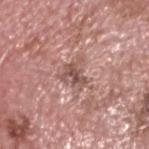No biopsy was performed on this lesion — it was imaged during a full skin examination and was not determined to be concerning. Measured at roughly 3.5 mm in maximum diameter. Imaged with white-light lighting. An algorithmic analysis of the crop reported an average lesion color of about L≈53 a*≈21 b*≈23 (CIELAB), about 10 CIELAB-L* units darker than the surrounding skin, and a normalized lesion–skin contrast near 7. The software also gave a nevus-likeness score of about 0/100 and a lesion-detection confidence of about 55/100. A male patient, aged approximately 75. A 15 mm crop from a total-body photograph taken for skin-cancer surveillance. The lesion is on the head or neck.